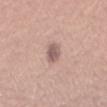  biopsy_status: not biopsied; imaged during a skin examination
  lighting: white-light
  lesion_size:
    long_diameter_mm_approx: 2.5
  automated_metrics:
    cielab_L: 58
    cielab_a: 18
    cielab_b: 21
    vs_skin_darker_L: 12.0
    vs_skin_contrast_norm: 8.0
    nevus_likeness_0_100: 5
    lesion_detection_confidence_0_100: 100
  site: arm
  patient:
    sex: female
    age_approx: 65
  image:
    source: total-body photography crop
    field_of_view_mm: 15